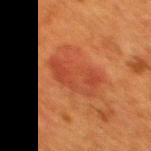Q: Was a biopsy performed?
A: imaged on a skin check; not biopsied
Q: What kind of image is this?
A: 15 mm crop, total-body photography
Q: Automated lesion metrics?
A: a lesion color around L≈39 a*≈28 b*≈33 in CIELAB
Q: What are the patient's age and sex?
A: female, in their 40s
Q: Where on the body is the lesion?
A: the back